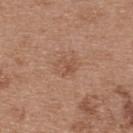Impression: Recorded during total-body skin imaging; not selected for excision or biopsy. Context: This is a white-light tile. A female subject, in their 40s. The lesion is on the upper back. The lesion's longest dimension is about 3 mm. An algorithmic analysis of the crop reported an area of roughly 5 mm², an eccentricity of roughly 0.65, and two-axis asymmetry of about 0.3. The software also gave a lesion color around L≈52 a*≈21 b*≈30 in CIELAB, a lesion–skin lightness drop of about 7, and a normalized lesion–skin contrast near 5. The software also gave a border-irregularity index near 3.5/10, a color-variation rating of about 4/10, and a peripheral color-asymmetry measure near 1.5. This image is a 15 mm lesion crop taken from a total-body photograph.